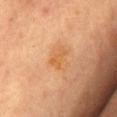workup = total-body-photography surveillance lesion; no biopsy | image = ~15 mm crop, total-body skin-cancer survey | body site = the chest | patient = female, aged 58 to 62.